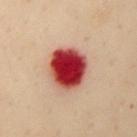Notes:
– workup — imaged on a skin check; not biopsied
– location — the chest
– patient — female, aged around 60
– diameter — ~5 mm (longest diameter)
– image-analysis metrics — a shape eccentricity near 0.45; about 22 CIELAB-L* units darker than the surrounding skin and a lesion-to-skin contrast of about 16 (normalized; higher = more distinct); a classifier nevus-likeness of about 0/100 and lesion-presence confidence of about 100/100
– tile lighting — cross-polarized
– acquisition — total-body-photography crop, ~15 mm field of view